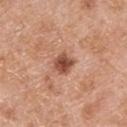Notes:
- acquisition · 15 mm crop, total-body photography
- subject · male, aged approximately 80
- automated metrics · an area of roughly 5.5 mm² and an outline eccentricity of about 0.4 (0 = round, 1 = elongated); a lesion color around L≈51 a*≈24 b*≈31 in CIELAB, about 14 CIELAB-L* units darker than the surrounding skin, and a lesion-to-skin contrast of about 9.5 (normalized; higher = more distinct)
- size · about 2.5 mm
- anatomic site · the left lower leg
- lighting · white-light